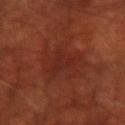Captured during whole-body skin photography for melanoma surveillance; the lesion was not biopsied. The tile uses cross-polarized illumination. Approximately 4.5 mm at its widest. A male subject, approximately 70 years of age. On the arm. A close-up tile cropped from a whole-body skin photograph, about 15 mm across.